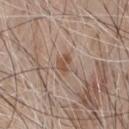No biopsy was performed on this lesion — it was imaged during a full skin examination and was not determined to be concerning.
A male patient, roughly 80 years of age.
On the front of the torso.
About 3 mm across.
A lesion tile, about 15 mm wide, cut from a 3D total-body photograph.
Automated image analysis of the tile measured a border-irregularity index near 2.5/10. It also reported an automated nevus-likeness rating near 0 out of 100 and a lesion-detection confidence of about 100/100.